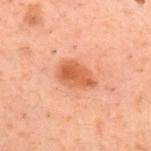The lesion was photographed on a routine skin check and not biopsied; there is no pathology result.